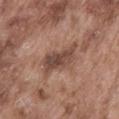{"biopsy_status": "not biopsied; imaged during a skin examination", "site": "abdomen", "lesion_size": {"long_diameter_mm_approx": 5.5}, "lighting": "white-light", "automated_metrics": {"area_mm2_approx": 10.0, "eccentricity": 0.9, "cielab_L": 46, "cielab_a": 19, "cielab_b": 25, "vs_skin_darker_L": 11.0, "nevus_likeness_0_100": 0, "lesion_detection_confidence_0_100": 95}, "patient": {"sex": "male", "age_approx": 75}, "image": {"source": "total-body photography crop", "field_of_view_mm": 15}}